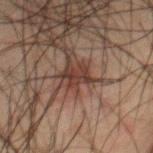The total-body-photography lesion software estimated a lesion–skin lightness drop of about 9 and a normalized border contrast of about 9. The software also gave a border-irregularity rating of about 7.5/10, a color-variation rating of about 1.5/10, and peripheral color asymmetry of about 0.5. The analysis additionally found an automated nevus-likeness rating near 25 out of 100 and a detector confidence of about 90 out of 100 that the crop contains a lesion.
A male patient, about 65 years old.
The tile uses cross-polarized illumination.
The lesion is on the chest.
About 3 mm across.
A lesion tile, about 15 mm wide, cut from a 3D total-body photograph.